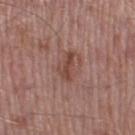Captured during whole-body skin photography for melanoma surveillance; the lesion was not biopsied. A region of skin cropped from a whole-body photographic capture, roughly 15 mm wide. A male subject, aged approximately 55. The lesion is located on the right thigh.